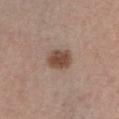The lesion was photographed on a routine skin check and not biopsied; there is no pathology result.
Cropped from a whole-body photographic skin survey; the tile spans about 15 mm.
The lesion is on the chest.
A male patient aged 38 to 42.